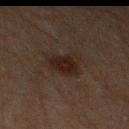Findings:
• workup · total-body-photography surveillance lesion; no biopsy
• acquisition · ~15 mm tile from a whole-body skin photo
• diameter · ≈3 mm
• automated lesion analysis · an automated nevus-likeness rating near 100 out of 100 and a detector confidence of about 100 out of 100 that the crop contains a lesion
• body site · the left thigh
• patient · male, aged approximately 60
• illumination · cross-polarized illumination